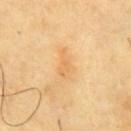Impression: Imaged during a routine full-body skin examination; the lesion was not biopsied and no histopathology is available. Acquisition and patient details: Captured under cross-polarized illumination. The recorded lesion diameter is about 3.5 mm. A male subject, in their mid- to late 60s. From the chest. A roughly 15 mm field-of-view crop from a total-body skin photograph.